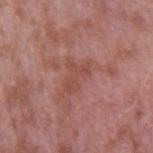Notes:
• workup — imaged on a skin check; not biopsied